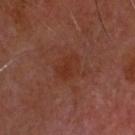Clinical impression: No biopsy was performed on this lesion — it was imaged during a full skin examination and was not determined to be concerning. Context: The recorded lesion diameter is about 3 mm. Cropped from a total-body skin-imaging series; the visible field is about 15 mm. The lesion is located on the head or neck. A male patient, roughly 60 years of age.